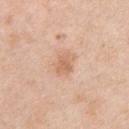Q: Was a biopsy performed?
A: catalogued during a skin exam; not biopsied
Q: Patient demographics?
A: female, roughly 40 years of age
Q: How was the tile lit?
A: white-light illumination
Q: What did automated image analysis measure?
A: border irregularity of about 2.5 on a 0–10 scale
Q: What is the imaging modality?
A: 15 mm crop, total-body photography
Q: Where on the body is the lesion?
A: the right upper arm
Q: Lesion size?
A: about 3 mm